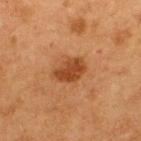<tbp_lesion>
<biopsy_status>not biopsied; imaged during a skin examination</biopsy_status>
</tbp_lesion>